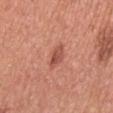| field | value |
|---|---|
| notes | no biopsy performed (imaged during a skin exam) |
| lighting | white-light illumination |
| image source | ~15 mm tile from a whole-body skin photo |
| size | ~3 mm (longest diameter) |
| patient | female, about 65 years old |
| site | the left upper arm |